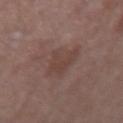| feature | finding |
|---|---|
| notes | catalogued during a skin exam; not biopsied |
| lesion size | ~4 mm (longest diameter) |
| acquisition | ~15 mm crop, total-body skin-cancer survey |
| lighting | white-light illumination |
| automated lesion analysis | border irregularity of about 3.5 on a 0–10 scale; a nevus-likeness score of about 0/100 and lesion-presence confidence of about 100/100 |
| subject | female, about 55 years old |
| body site | the arm |